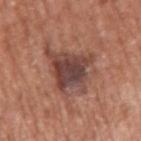Assessment:
Part of a total-body skin-imaging series; this lesion was reviewed on a skin check and was not flagged for biopsy.
Clinical summary:
Cropped from a total-body skin-imaging series; the visible field is about 15 mm. Automated image analysis of the tile measured a lesion area of about 23 mm², a shape eccentricity near 0.7, and two-axis asymmetry of about 0.4. And it measured a mean CIELAB color near L≈45 a*≈21 b*≈24 and a normalized border contrast of about 9. The analysis additionally found a border-irregularity index near 6.5/10, a within-lesion color-variation index near 7.5/10, and a peripheral color-asymmetry measure near 2. It also reported an automated nevus-likeness rating near 20 out of 100 and lesion-presence confidence of about 100/100. The lesion's longest dimension is about 8 mm. Located on the right upper arm. The patient is a male approximately 75 years of age. The tile uses white-light illumination.